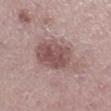Captured during whole-body skin photography for melanoma surveillance; the lesion was not biopsied.
The lesion is on the right lower leg.
Captured under white-light illumination.
A 15 mm close-up tile from a total-body photography series done for melanoma screening.
Longest diameter approximately 6.5 mm.
A female subject, aged approximately 40.